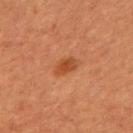Impression: The lesion was tiled from a total-body skin photograph and was not biopsied. Clinical summary: The lesion is on the mid back. Imaged with cross-polarized lighting. Cropped from a total-body skin-imaging series; the visible field is about 15 mm. A male subject, aged 48 to 52. The lesion's longest dimension is about 3 mm.